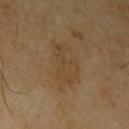The lesion was tiled from a total-body skin photograph and was not biopsied. The lesion is located on the left upper arm. A male patient, aged approximately 70. Longest diameter approximately 5.5 mm. A 15 mm close-up extracted from a 3D total-body photography capture. The tile uses cross-polarized illumination.